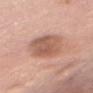| key | value |
|---|---|
| notes | total-body-photography surveillance lesion; no biopsy |
| diameter | ≈6 mm |
| acquisition | total-body-photography crop, ~15 mm field of view |
| tile lighting | white-light |
| subject | female, roughly 60 years of age |
| anatomic site | the chest |